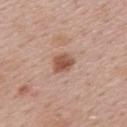Part of a total-body skin-imaging series; this lesion was reviewed on a skin check and was not flagged for biopsy. An algorithmic analysis of the crop reported a shape-asymmetry score of about 0.25 (0 = symmetric). The analysis additionally found an average lesion color of about L≈53 a*≈21 b*≈29 (CIELAB), a lesion–skin lightness drop of about 12, and a normalized border contrast of about 8.5. The analysis additionally found a classifier nevus-likeness of about 90/100. A 15 mm crop from a total-body photograph taken for skin-cancer surveillance. A male subject, aged around 55. Captured under white-light illumination. The lesion is on the back.